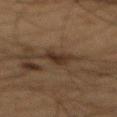No biopsy was performed on this lesion — it was imaged during a full skin examination and was not determined to be concerning. The lesion is located on the mid back. A 15 mm close-up extracted from a 3D total-body photography capture. This is a cross-polarized tile. A male subject, aged approximately 65.